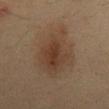| field | value |
|---|---|
| biopsy status | imaged on a skin check; not biopsied |
| subject | male, aged approximately 35 |
| anatomic site | the mid back |
| lesion diameter | ~8 mm (longest diameter) |
| image source | ~15 mm tile from a whole-body skin photo |
| illumination | cross-polarized illumination |